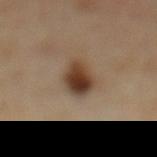The lesion was photographed on a routine skin check and not biopsied; there is no pathology result.
Located on the mid back.
A male subject aged 63 to 67.
A lesion tile, about 15 mm wide, cut from a 3D total-body photograph.
The tile uses cross-polarized illumination.
An algorithmic analysis of the crop reported a classifier nevus-likeness of about 100/100 and a lesion-detection confidence of about 100/100.